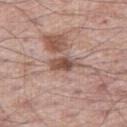biopsy status = no biopsy performed (imaged during a skin exam) | lesion diameter = about 3.5 mm | tile lighting = white-light | location = the right thigh | imaging modality = total-body-photography crop, ~15 mm field of view | subject = male, roughly 60 years of age.